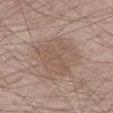The lesion was tiled from a total-body skin photograph and was not biopsied.
A male subject, aged around 35.
Longest diameter approximately 5.5 mm.
Cropped from a whole-body photographic skin survey; the tile spans about 15 mm.
The lesion-visualizer software estimated an area of roughly 20 mm², an outline eccentricity of about 0.6 (0 = round, 1 = elongated), and a shape-asymmetry score of about 0.25 (0 = symmetric). The analysis additionally found a lesion color around L≈54 a*≈16 b*≈25 in CIELAB, a lesion–skin lightness drop of about 7, and a lesion-to-skin contrast of about 5 (normalized; higher = more distinct). And it measured internal color variation of about 2.5 on a 0–10 scale.
Located on the left lower leg.